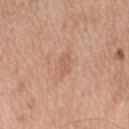No biopsy was performed on this lesion — it was imaged during a full skin examination and was not determined to be concerning. A 15 mm crop from a total-body photograph taken for skin-cancer surveillance. This is a white-light tile. An algorithmic analysis of the crop reported a lesion area of about 4 mm², a shape eccentricity near 0.8, and two-axis asymmetry of about 0.25. It also reported a lesion color around L≈60 a*≈21 b*≈31 in CIELAB and a lesion–skin lightness drop of about 6. The analysis additionally found a border-irregularity rating of about 3/10 and peripheral color asymmetry of about 0.5. It also reported an automated nevus-likeness rating near 0 out of 100. From the mid back. The subject is a male aged 73–77. The lesion's longest dimension is about 3 mm.